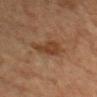Q: Was a biopsy performed?
A: imaged on a skin check; not biopsied
Q: What is the anatomic site?
A: the head or neck
Q: Lesion size?
A: about 4 mm
Q: Patient demographics?
A: male, in their mid- to late 40s
Q: Illumination type?
A: cross-polarized
Q: What did automated image analysis measure?
A: an average lesion color of about L≈34 a*≈18 b*≈28 (CIELAB) and a normalized lesion–skin contrast near 7.5
Q: What is the imaging modality?
A: ~15 mm crop, total-body skin-cancer survey